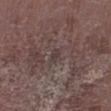Q: Was this lesion biopsied?
A: no biopsy performed (imaged during a skin exam)
Q: How was this image acquired?
A: ~15 mm crop, total-body skin-cancer survey
Q: What is the lesion's diameter?
A: about 2 mm
Q: What are the patient's age and sex?
A: male, in their mid-70s
Q: What is the anatomic site?
A: the leg
Q: Illumination type?
A: white-light